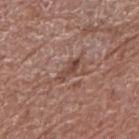The tile uses white-light illumination.
Automated tile analysis of the lesion measured a footprint of about 3.5 mm², an outline eccentricity of about 0.9 (0 = round, 1 = elongated), and a symmetry-axis asymmetry near 0.3. The software also gave a lesion color around L≈45 a*≈20 b*≈25 in CIELAB. And it measured an automated nevus-likeness rating near 0 out of 100.
The lesion is on the right upper arm.
Measured at roughly 3.5 mm in maximum diameter.
The subject is a male roughly 70 years of age.
This image is a 15 mm lesion crop taken from a total-body photograph.